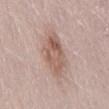Clinical summary:
Longest diameter approximately 6.5 mm. Cropped from a whole-body photographic skin survey; the tile spans about 15 mm. Automated image analysis of the tile measured a footprint of about 14 mm², an eccentricity of roughly 0.9, and two-axis asymmetry of about 0.2. The analysis additionally found a lesion color around L≈58 a*≈18 b*≈25 in CIELAB and a lesion–skin lightness drop of about 11. The analysis additionally found a border-irregularity index near 3/10 and peripheral color asymmetry of about 2. It also reported an automated nevus-likeness rating near 15 out of 100 and a lesion-detection confidence of about 100/100. This is a white-light tile. A female subject in their 30s. Located on the lower back.